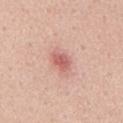Captured during whole-body skin photography for melanoma surveillance; the lesion was not biopsied. From the mid back. The lesion's longest dimension is about 3 mm. This is a white-light tile. Automated tile analysis of the lesion measured a symmetry-axis asymmetry near 0.25. The software also gave a mean CIELAB color near L≈61 a*≈27 b*≈26, roughly 12 lightness units darker than nearby skin, and a lesion-to-skin contrast of about 7 (normalized; higher = more distinct). And it measured a nevus-likeness score of about 20/100. The subject is a male approximately 55 years of age. A 15 mm close-up extracted from a 3D total-body photography capture.